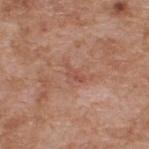The subject is a male aged 58–62.
The tile uses white-light illumination.
The lesion is on the upper back.
The total-body-photography lesion software estimated a lesion area of about 2.5 mm², an outline eccentricity of about 0.9 (0 = round, 1 = elongated), and two-axis asymmetry of about 0.45. It also reported an average lesion color of about L≈51 a*≈24 b*≈29 (CIELAB), a lesion–skin lightness drop of about 7, and a normalized border contrast of about 5. The analysis additionally found border irregularity of about 4.5 on a 0–10 scale, internal color variation of about 0 on a 0–10 scale, and peripheral color asymmetry of about 0. It also reported an automated nevus-likeness rating near 0 out of 100.
This image is a 15 mm lesion crop taken from a total-body photograph.
The lesion's longest dimension is about 2.5 mm.